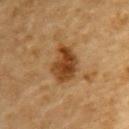<record>
<biopsy_status>not biopsied; imaged during a skin examination</biopsy_status>
<patient>
  <sex>male</sex>
  <age_approx>85+</age_approx>
</patient>
<image>
  <source>total-body photography crop</source>
  <field_of_view_mm>15</field_of_view_mm>
</image>
<site>upper back</site>
</record>